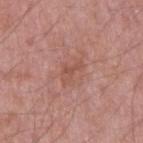Clinical impression: Recorded during total-body skin imaging; not selected for excision or biopsy. Acquisition and patient details: The subject is a male aged around 50. About 3.5 mm across. This image is a 15 mm lesion crop taken from a total-body photograph. Imaged with white-light lighting. Located on the right upper arm.